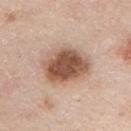Recorded during total-body skin imaging; not selected for excision or biopsy.
A roughly 15 mm field-of-view crop from a total-body skin photograph.
The subject is a male aged 43 to 47.
From the chest.
Imaged with white-light lighting.
Automated tile analysis of the lesion measured a lesion area of about 18 mm² and an outline eccentricity of about 0.6 (0 = round, 1 = elongated). And it measured a lesion color around L≈54 a*≈20 b*≈29 in CIELAB, a lesion–skin lightness drop of about 17, and a normalized border contrast of about 11. It also reported border irregularity of about 1.5 on a 0–10 scale and a peripheral color-asymmetry measure near 1.5. The software also gave a classifier nevus-likeness of about 90/100 and lesion-presence confidence of about 100/100.